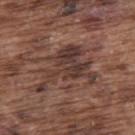Case summary:
- follow-up — no biopsy performed (imaged during a skin exam)
- patient — male, roughly 75 years of age
- image — total-body-photography crop, ~15 mm field of view
- tile lighting — white-light illumination
- anatomic site — the upper back